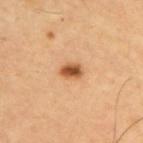biopsy status — no biopsy performed (imaged during a skin exam) | body site — the upper back | lesion size — about 2.5 mm | acquisition — ~15 mm tile from a whole-body skin photo | subject — male, aged 63–67 | illumination — cross-polarized illumination.